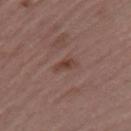follow-up: no biopsy performed (imaged during a skin exam)
image source: 15 mm crop, total-body photography
subject: male, aged 63 to 67
size: ~2.5 mm (longest diameter)
body site: the leg
tile lighting: white-light illumination